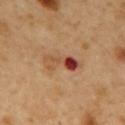Clinical impression:
Recorded during total-body skin imaging; not selected for excision or biopsy.
Image and clinical context:
This is a cross-polarized tile. Approximately 4 mm at its widest. An algorithmic analysis of the crop reported a shape eccentricity near 0.9 and two-axis asymmetry of about 0.4. A male patient aged approximately 50. The lesion is on the mid back. A lesion tile, about 15 mm wide, cut from a 3D total-body photograph.